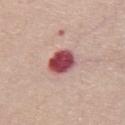Q: Is there a histopathology result?
A: catalogued during a skin exam; not biopsied
Q: What kind of image is this?
A: total-body-photography crop, ~15 mm field of view
Q: Lesion location?
A: the front of the torso
Q: What are the patient's age and sex?
A: female, aged approximately 70